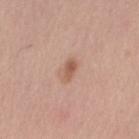Q: Was a biopsy performed?
A: no biopsy performed (imaged during a skin exam)
Q: Patient demographics?
A: male, aged approximately 60
Q: How was the tile lit?
A: white-light
Q: Lesion size?
A: about 2.5 mm
Q: What is the anatomic site?
A: the right upper arm
Q: Automated lesion metrics?
A: border irregularity of about 2.5 on a 0–10 scale and internal color variation of about 1.5 on a 0–10 scale; a nevus-likeness score of about 75/100 and a lesion-detection confidence of about 100/100
Q: What kind of image is this?
A: total-body-photography crop, ~15 mm field of view